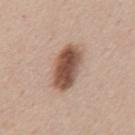biopsy_status: not biopsied; imaged during a skin examination
patient:
  sex: male
  age_approx: 45
image:
  source: total-body photography crop
  field_of_view_mm: 15
site: mid back
lesion_size:
  long_diameter_mm_approx: 5.5